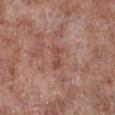follow-up — imaged on a skin check; not biopsied | diameter — ≈3 mm | illumination — white-light | image — total-body-photography crop, ~15 mm field of view | location — the right lower leg | subject — male, in their mid- to late 50s.